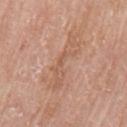Impression: No biopsy was performed on this lesion — it was imaged during a full skin examination and was not determined to be concerning. Background: Captured under white-light illumination. The lesion is on the left upper arm. A female patient, aged around 65. Longest diameter approximately 6.5 mm. A 15 mm close-up tile from a total-body photography series done for melanoma screening.